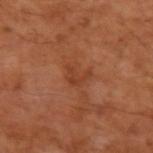Clinical summary:
Measured at roughly 3 mm in maximum diameter. The lesion-visualizer software estimated an area of roughly 3.5 mm², an eccentricity of roughly 0.65, and a symmetry-axis asymmetry near 0.65. And it measured a border-irregularity index near 7/10, internal color variation of about 1 on a 0–10 scale, and a peripheral color-asymmetry measure near 0.5. The software also gave an automated nevus-likeness rating near 0 out of 100 and a detector confidence of about 100 out of 100 that the crop contains a lesion. Captured under cross-polarized illumination. A region of skin cropped from a whole-body photographic capture, roughly 15 mm wide. On the left upper arm. The subject is a male aged approximately 55.